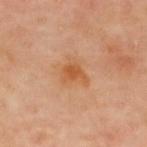Impression: The lesion was tiled from a total-body skin photograph and was not biopsied. Acquisition and patient details: A roughly 15 mm field-of-view crop from a total-body skin photograph. The total-body-photography lesion software estimated a lesion area of about 5.5 mm², a shape eccentricity near 0.6, and two-axis asymmetry of about 0.45. The software also gave a lesion color around L≈56 a*≈25 b*≈40 in CIELAB and roughly 8 lightness units darker than nearby skin. The analysis additionally found a classifier nevus-likeness of about 70/100. A male patient aged around 65. The lesion is on the upper back. Longest diameter approximately 3.5 mm.